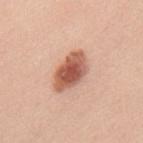Q: Illumination type?
A: white-light illumination
Q: What is the anatomic site?
A: the upper back
Q: How was this image acquired?
A: ~15 mm tile from a whole-body skin photo
Q: Patient demographics?
A: female, aged 38–42
Q: What did automated image analysis measure?
A: a lesion area of about 11 mm², an eccentricity of roughly 0.85, and a shape-asymmetry score of about 0.2 (0 = symmetric); an automated nevus-likeness rating near 100 out of 100 and a lesion-detection confidence of about 100/100
Q: What is the lesion's diameter?
A: about 5 mm